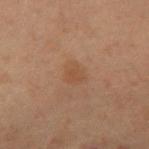Impression:
The lesion was photographed on a routine skin check and not biopsied; there is no pathology result.
Background:
A male subject aged 58–62. The lesion is on the back. A 15 mm close-up tile from a total-body photography series done for melanoma screening.